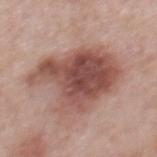biopsy status: imaged on a skin check; not biopsied
acquisition: 15 mm crop, total-body photography
location: the mid back
lighting: white-light
subject: male, in their mid-60s
lesion size: ~9 mm (longest diameter)
automated lesion analysis: an average lesion color of about L≈50 a*≈22 b*≈24 (CIELAB), about 14 CIELAB-L* units darker than the surrounding skin, and a normalized border contrast of about 10; a border-irregularity index near 4/10, internal color variation of about 7 on a 0–10 scale, and peripheral color asymmetry of about 2.5; a nevus-likeness score of about 85/100 and a lesion-detection confidence of about 100/100